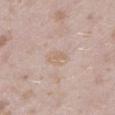notes = catalogued during a skin exam; not biopsied
location = the left lower leg
patient = female, roughly 25 years of age
imaging modality = ~15 mm tile from a whole-body skin photo